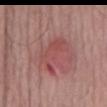This lesion was catalogued during total-body skin photography and was not selected for biopsy. Cropped from a total-body skin-imaging series; the visible field is about 15 mm. From the mid back. The total-body-photography lesion software estimated an area of roughly 9 mm², a shape eccentricity near 0.85, and a symmetry-axis asymmetry near 0.55. It also reported an average lesion color of about L≈49 a*≈28 b*≈23 (CIELAB) and a lesion–skin lightness drop of about 7. And it measured an automated nevus-likeness rating near 0 out of 100. The subject is a male aged approximately 65. Longest diameter approximately 5 mm.